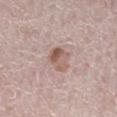notes: imaged on a skin check; not biopsied
tile lighting: white-light
automated metrics: a lesion area of about 6 mm², a shape eccentricity near 0.5, and a shape-asymmetry score of about 0.25 (0 = symmetric); a lesion color around L≈57 a*≈18 b*≈24 in CIELAB, a lesion–skin lightness drop of about 10, and a normalized border contrast of about 7.5; border irregularity of about 2.5 on a 0–10 scale and radial color variation of about 3; a nevus-likeness score of about 45/100 and a lesion-detection confidence of about 100/100
patient: male, aged around 75
location: the left lower leg
image: ~15 mm tile from a whole-body skin photo
lesion diameter: about 3 mm